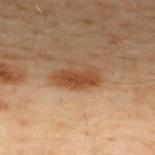Impression:
The lesion was tiled from a total-body skin photograph and was not biopsied.